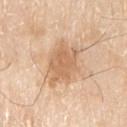Recorded during total-body skin imaging; not selected for excision or biopsy. Automated image analysis of the tile measured a footprint of about 14 mm². The analysis additionally found a mean CIELAB color near L≈65 a*≈19 b*≈36 and a normalized border contrast of about 7. A lesion tile, about 15 mm wide, cut from a 3D total-body photograph. A male patient approximately 80 years of age. About 5.5 mm across. Captured under white-light illumination. The lesion is located on the left upper arm.